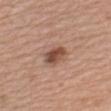Q: What is the imaging modality?
A: 15 mm crop, total-body photography
Q: Patient demographics?
A: male, aged around 60
Q: Lesion location?
A: the left upper arm